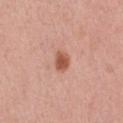The lesion is located on the abdomen.
A female patient, aged around 40.
This is a white-light tile.
Longest diameter approximately 2.5 mm.
A 15 mm close-up tile from a total-body photography series done for melanoma screening.